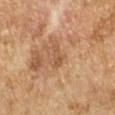Impression:
Recorded during total-body skin imaging; not selected for excision or biopsy.
Acquisition and patient details:
The lesion is located on the left forearm. Measured at roughly 3 mm in maximum diameter. The total-body-photography lesion software estimated an outline eccentricity of about 0.95 (0 = round, 1 = elongated). The analysis additionally found an average lesion color of about L≈46 a*≈19 b*≈30 (CIELAB), roughly 7 lightness units darker than nearby skin, and a normalized lesion–skin contrast near 5.5. A female patient, aged 58 to 62. A lesion tile, about 15 mm wide, cut from a 3D total-body photograph.